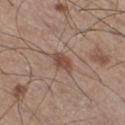Q: Was a biopsy performed?
A: total-body-photography surveillance lesion; no biopsy
Q: Illumination type?
A: white-light illumination
Q: Where on the body is the lesion?
A: the right thigh
Q: What are the patient's age and sex?
A: male, roughly 60 years of age
Q: What is the lesion's diameter?
A: about 3 mm
Q: Automated lesion metrics?
A: an average lesion color of about L≈47 a*≈18 b*≈25 (CIELAB), roughly 10 lightness units darker than nearby skin, and a normalized lesion–skin contrast near 7.5; a border-irregularity rating of about 2.5/10 and peripheral color asymmetry of about 0.5
Q: How was this image acquired?
A: total-body-photography crop, ~15 mm field of view